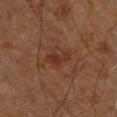Impression:
Captured during whole-body skin photography for melanoma surveillance; the lesion was not biopsied.
Context:
The lesion-visualizer software estimated a lesion color around L≈23 a*≈18 b*≈23 in CIELAB, about 5 CIELAB-L* units darker than the surrounding skin, and a normalized border contrast of about 6. This is a cross-polarized tile. The subject is a male roughly 60 years of age. The lesion is on the chest. About 3 mm across. A 15 mm close-up extracted from a 3D total-body photography capture.